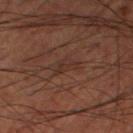{
  "biopsy_status": "not biopsied; imaged during a skin examination",
  "image": {
    "source": "total-body photography crop",
    "field_of_view_mm": 15
  },
  "patient": {
    "sex": "male",
    "age_approx": 60
  },
  "site": "right thigh"
}